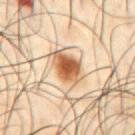Cropped from a whole-body photographic skin survey; the tile spans about 15 mm.
The lesion is on the abdomen.
The tile uses cross-polarized illumination.
A male patient approximately 50 years of age.
The recorded lesion diameter is about 4.5 mm.
Automated image analysis of the tile measured an eccentricity of roughly 0.7 and a shape-asymmetry score of about 0.2 (0 = symmetric).